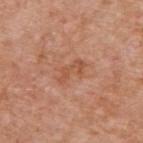biopsy status=catalogued during a skin exam; not biopsied
imaging modality=~15 mm tile from a whole-body skin photo
patient=male, aged 58–62
diameter=~3.5 mm (longest diameter)
site=the upper back